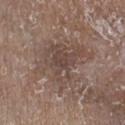No biopsy was performed on this lesion — it was imaged during a full skin examination and was not determined to be concerning. The lesion is on the right lower leg. The patient is a male roughly 65 years of age. A close-up tile cropped from a whole-body skin photograph, about 15 mm across.